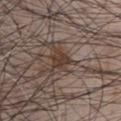  biopsy_status: not biopsied; imaged during a skin examination
  automated_metrics:
    eccentricity: 0.55
    nevus_likeness_0_100: 70
    lesion_detection_confidence_0_100: 95
  image:
    source: total-body photography crop
    field_of_view_mm: 15
  site: chest
  lighting: white-light
  lesion_size:
    long_diameter_mm_approx: 3.0
  patient:
    sex: male
    age_approx: 65